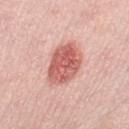The lesion was tiled from a total-body skin photograph and was not biopsied. This is a white-light tile. Cropped from a whole-body photographic skin survey; the tile spans about 15 mm. Longest diameter approximately 6 mm. A female patient aged approximately 65. On the left thigh. Automated tile analysis of the lesion measured an eccentricity of roughly 0.8 and a shape-asymmetry score of about 0.15 (0 = symmetric). The software also gave an average lesion color of about L≈61 a*≈29 b*≈27 (CIELAB) and roughly 14 lightness units darker than nearby skin. And it measured a border-irregularity rating of about 1.5/10 and a peripheral color-asymmetry measure near 1.5. It also reported a classifier nevus-likeness of about 95/100 and lesion-presence confidence of about 100/100.